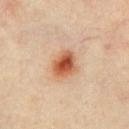Part of a total-body skin-imaging series; this lesion was reviewed on a skin check and was not flagged for biopsy. The total-body-photography lesion software estimated an average lesion color of about L≈56 a*≈25 b*≈35 (CIELAB), about 14 CIELAB-L* units darker than the surrounding skin, and a normalized border contrast of about 10. And it measured a border-irregularity rating of about 2/10, a within-lesion color-variation index near 8/10, and radial color variation of about 2. The lesion is on the chest. Cropped from a total-body skin-imaging series; the visible field is about 15 mm. A female subject, in their mid- to late 40s. Imaged with cross-polarized lighting.